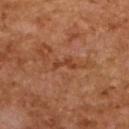workup = total-body-photography surveillance lesion; no biopsy
anatomic site = the upper back
tile lighting = cross-polarized illumination
lesion size = ≈3.5 mm
patient = female, aged around 60
imaging modality = total-body-photography crop, ~15 mm field of view
image-analysis metrics = a border-irregularity index near 6.5/10, internal color variation of about 0 on a 0–10 scale, and peripheral color asymmetry of about 0; a classifier nevus-likeness of about 0/100 and lesion-presence confidence of about 75/100